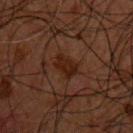Part of a total-body skin-imaging series; this lesion was reviewed on a skin check and was not flagged for biopsy.
A close-up tile cropped from a whole-body skin photograph, about 15 mm across.
A male patient roughly 50 years of age.
On the chest.
Captured under cross-polarized illumination.